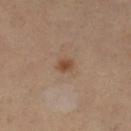Captured during whole-body skin photography for melanoma surveillance; the lesion was not biopsied.
A female subject, roughly 40 years of age.
A region of skin cropped from a whole-body photographic capture, roughly 15 mm wide.
Located on the left lower leg.